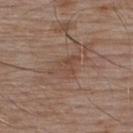Part of a total-body skin-imaging series; this lesion was reviewed on a skin check and was not flagged for biopsy. Imaged with white-light lighting. Located on the upper back. About 4 mm across. A male patient roughly 55 years of age. A 15 mm close-up tile from a total-body photography series done for melanoma screening.